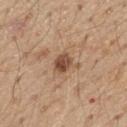follow-up: catalogued during a skin exam; not biopsied
automated metrics: a border-irregularity rating of about 2.5/10, internal color variation of about 4 on a 0–10 scale, and a peripheral color-asymmetry measure near 1.5
site: the chest
subject: male, aged around 70
image: ~15 mm crop, total-body skin-cancer survey
lighting: white-light illumination
lesion diameter: ~2.5 mm (longest diameter)